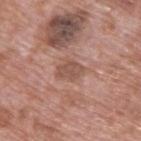<case>
  <patient>
    <sex>male</sex>
    <age_approx>70</age_approx>
  </patient>
  <lesion_size>
    <long_diameter_mm_approx>3.0</long_diameter_mm_approx>
  </lesion_size>
  <image>
    <source>total-body photography crop</source>
    <field_of_view_mm>15</field_of_view_mm>
  </image>
  <automated_metrics>
    <border_irregularity_0_10>2.5</border_irregularity_0_10>
    <color_variation_0_10>1.5</color_variation_0_10>
    <peripheral_color_asymmetry>0.5</peripheral_color_asymmetry>
  </automated_metrics>
  <site>upper back</site>
</case>